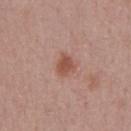{"biopsy_status": "not biopsied; imaged during a skin examination", "site": "front of the torso", "patient": {"sex": "male", "age_approx": 45}, "image": {"source": "total-body photography crop", "field_of_view_mm": 15}}